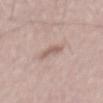TBP lesion metrics: an outline eccentricity of about 0.9 (0 = round, 1 = elongated); a border-irregularity index near 3/10, internal color variation of about 1 on a 0–10 scale, and a peripheral color-asymmetry measure near 0.5
subject: male, approximately 55 years of age
lighting: white-light
acquisition: ~15 mm crop, total-body skin-cancer survey